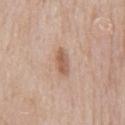No biopsy was performed on this lesion — it was imaged during a full skin examination and was not determined to be concerning. Approximately 4 mm at its widest. From the front of the torso. Cropped from a total-body skin-imaging series; the visible field is about 15 mm. A male patient approximately 65 years of age. This is a white-light tile.